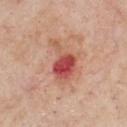Imaged during a routine full-body skin examination; the lesion was not biopsied and no histopathology is available. A 15 mm close-up extracted from a 3D total-body photography capture. Located on the chest. A male subject, aged around 55. This is a white-light tile. The total-body-photography lesion software estimated an eccentricity of roughly 0.75 and a symmetry-axis asymmetry near 0.45. It also reported border irregularity of about 5 on a 0–10 scale, a color-variation rating of about 9/10, and peripheral color asymmetry of about 3.